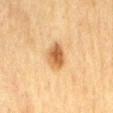Context:
Measured at roughly 3.5 mm in maximum diameter. The subject is a female aged 53–57. A 15 mm close-up extracted from a 3D total-body photography capture. Captured under cross-polarized illumination. The lesion is located on the mid back.